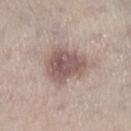biopsy_status: not biopsied; imaged during a skin examination
lesion_size:
  long_diameter_mm_approx: 5.0
site: right lower leg
patient:
  sex: male
  age_approx: 60
image:
  source: total-body photography crop
  field_of_view_mm: 15
lighting: white-light